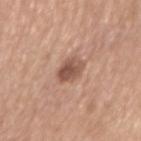notes = total-body-photography surveillance lesion; no biopsy | image source = 15 mm crop, total-body photography | diameter = about 3 mm | body site = the arm | subject = female, aged 48 to 52.